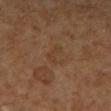Clinical impression:
Captured during whole-body skin photography for melanoma surveillance; the lesion was not biopsied.
Background:
Located on the right lower leg. A roughly 15 mm field-of-view crop from a total-body skin photograph. The patient is a female approximately 65 years of age. Measured at roughly 3 mm in maximum diameter.